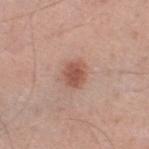follow-up: no biopsy performed (imaged during a skin exam) | tile lighting: white-light | acquisition: ~15 mm tile from a whole-body skin photo | location: the left lower leg | subject: male, aged approximately 50 | automated metrics: an area of roughly 5 mm² and a symmetry-axis asymmetry near 0.2; a mean CIELAB color near L≈53 a*≈24 b*≈28, a lesion–skin lightness drop of about 12, and a normalized lesion–skin contrast near 8.